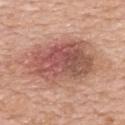| feature | finding |
|---|---|
| biopsy status | no biopsy performed (imaged during a skin exam) |
| acquisition | total-body-photography crop, ~15 mm field of view |
| anatomic site | the upper back |
| automated metrics | internal color variation of about 8 on a 0–10 scale and peripheral color asymmetry of about 2.5 |
| patient | female, approximately 60 years of age |